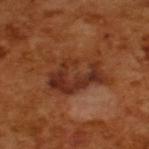Clinical impression: No biopsy was performed on this lesion — it was imaged during a full skin examination and was not determined to be concerning. Clinical summary: The subject is a male aged 63–67. The tile uses cross-polarized illumination. This image is a 15 mm lesion crop taken from a total-body photograph.